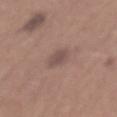Q: Is there a histopathology result?
A: no biopsy performed (imaged during a skin exam)
Q: Automated lesion metrics?
A: a lesion color around L≈49 a*≈17 b*≈21 in CIELAB, about 8 CIELAB-L* units darker than the surrounding skin, and a normalized border contrast of about 6.5
Q: What is the lesion's diameter?
A: ~3 mm (longest diameter)
Q: Who is the patient?
A: male, aged around 65
Q: Illumination type?
A: white-light illumination
Q: What is the imaging modality?
A: 15 mm crop, total-body photography
Q: Where on the body is the lesion?
A: the mid back